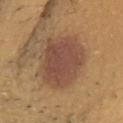Findings:
• biopsy status · no biopsy performed (imaged during a skin exam)
• lighting · white-light illumination
• patient · female, in their mid-20s
• anatomic site · the chest
• diameter · ~7 mm (longest diameter)
• TBP lesion metrics · an eccentricity of roughly 0.6 and two-axis asymmetry of about 0.15; a lesion color around L≈48 a*≈19 b*≈29 in CIELAB and a normalized border contrast of about 9; a color-variation rating of about 4/10 and peripheral color asymmetry of about 1
• imaging modality · total-body-photography crop, ~15 mm field of view